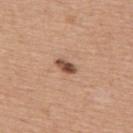notes = catalogued during a skin exam; not biopsied
imaging modality = 15 mm crop, total-body photography
illumination = white-light
subject = male, in their mid- to late 50s
body site = the back